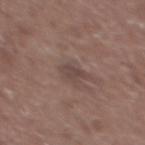• follow-up: total-body-photography surveillance lesion; no biopsy
• subject: male, approximately 60 years of age
• illumination: white-light
• location: the upper back
• image: total-body-photography crop, ~15 mm field of view
• lesion diameter: ~3 mm (longest diameter)
• automated lesion analysis: a classifier nevus-likeness of about 0/100